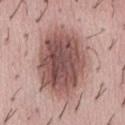Q: Was this lesion biopsied?
A: catalogued during a skin exam; not biopsied
Q: What is the imaging modality?
A: total-body-photography crop, ~15 mm field of view
Q: How was the tile lit?
A: white-light illumination
Q: What are the patient's age and sex?
A: female, aged around 40
Q: What is the anatomic site?
A: the abdomen